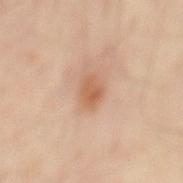* illumination — cross-polarized illumination
* image — 15 mm crop, total-body photography
* patient — about 55 years old
* site — the mid back
* lesion size — ≈3 mm